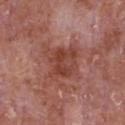biopsy status=catalogued during a skin exam; not biopsied | lesion size=about 4 mm | anatomic site=the front of the torso | lighting=white-light | image=~15 mm tile from a whole-body skin photo | patient=male, in their mid- to late 60s.